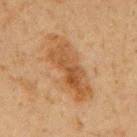Part of a total-body skin-imaging series; this lesion was reviewed on a skin check and was not flagged for biopsy. From the mid back. A male subject, aged around 60. This is a cross-polarized tile. Cropped from a whole-body photographic skin survey; the tile spans about 15 mm.